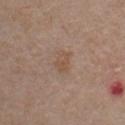workup — imaged on a skin check; not biopsied
image source — ~15 mm crop, total-body skin-cancer survey
body site — the chest
patient — male, approximately 55 years of age